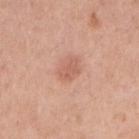Case summary:
- follow-up: imaged on a skin check; not biopsied
- location: the right upper arm
- patient: female, aged around 40
- size: ≈2.5 mm
- imaging modality: total-body-photography crop, ~15 mm field of view